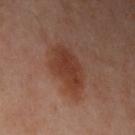biopsy_status: not biopsied; imaged during a skin examination
image:
  source: total-body photography crop
  field_of_view_mm: 15
lesion_size:
  long_diameter_mm_approx: 5.5
lighting: cross-polarized
site: left upper arm
patient:
  sex: female
  age_approx: 40